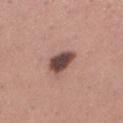Q: Was this lesion biopsied?
A: no biopsy performed (imaged during a skin exam)
Q: What kind of image is this?
A: 15 mm crop, total-body photography
Q: Where on the body is the lesion?
A: the left lower leg
Q: Patient demographics?
A: female, aged approximately 30